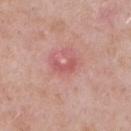Q: Is there a histopathology result?
A: imaged on a skin check; not biopsied
Q: Lesion size?
A: ≈3 mm
Q: How was the tile lit?
A: white-light illumination
Q: What kind of image is this?
A: 15 mm crop, total-body photography
Q: Lesion location?
A: the back
Q: Patient demographics?
A: male, aged around 55
Q: What did automated image analysis measure?
A: a shape eccentricity near 0.8 and a shape-asymmetry score of about 0.5 (0 = symmetric); roughly 8 lightness units darker than nearby skin; a color-variation rating of about 0.5/10 and a peripheral color-asymmetry measure near 0; a classifier nevus-likeness of about 0/100 and lesion-presence confidence of about 100/100